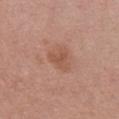{"biopsy_status": "not biopsied; imaged during a skin examination"}